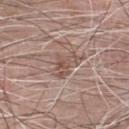{"biopsy_status": "not biopsied; imaged during a skin examination", "image": {"source": "total-body photography crop", "field_of_view_mm": 15}, "patient": {"sex": "male", "age_approx": 60}, "lesion_size": {"long_diameter_mm_approx": 3.5}, "site": "chest", "lighting": "white-light"}